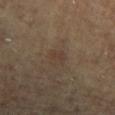Part of a total-body skin-imaging series; this lesion was reviewed on a skin check and was not flagged for biopsy.
An algorithmic analysis of the crop reported a lesion area of about 4 mm², an eccentricity of roughly 0.75, and a shape-asymmetry score of about 0.3 (0 = symmetric). And it measured a border-irregularity index near 3/10, internal color variation of about 1.5 on a 0–10 scale, and peripheral color asymmetry of about 0.5. The analysis additionally found a detector confidence of about 100 out of 100 that the crop contains a lesion.
The subject is a female aged approximately 80.
The tile uses cross-polarized illumination.
From the chest.
A 15 mm close-up tile from a total-body photography series done for melanoma screening.